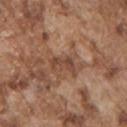Assessment:
This lesion was catalogued during total-body skin photography and was not selected for biopsy.
Image and clinical context:
From the left upper arm. This image is a 15 mm lesion crop taken from a total-body photograph. The recorded lesion diameter is about 3.5 mm. The total-body-photography lesion software estimated a lesion area of about 4 mm², an outline eccentricity of about 0.85 (0 = round, 1 = elongated), and two-axis asymmetry of about 0.45. It also reported a lesion color around L≈45 a*≈20 b*≈29 in CIELAB, a lesion–skin lightness drop of about 9, and a normalized border contrast of about 7. The tile uses white-light illumination. A male subject, in their mid- to late 70s.